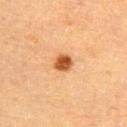Image and clinical context: Located on the back. The lesion's longest dimension is about 2.5 mm. A lesion tile, about 15 mm wide, cut from a 3D total-body photograph. A female subject in their mid- to late 60s. Captured under cross-polarized illumination. Automated tile analysis of the lesion measured a lesion–skin lightness drop of about 16 and a normalized border contrast of about 11.5. The analysis additionally found a color-variation rating of about 3/10 and a peripheral color-asymmetry measure near 1. The software also gave a classifier nevus-likeness of about 100/100 and a detector confidence of about 100 out of 100 that the crop contains a lesion.